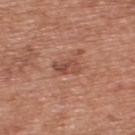Clinical impression:
No biopsy was performed on this lesion — it was imaged during a full skin examination and was not determined to be concerning.
Context:
The lesion is located on the back. A lesion tile, about 15 mm wide, cut from a 3D total-body photograph. A male patient approximately 70 years of age. The recorded lesion diameter is about 3 mm.